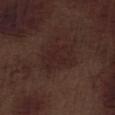| key | value |
|---|---|
| image | total-body-photography crop, ~15 mm field of view |
| patient | male, about 70 years old |
| lesion size | about 4.5 mm |
| site | the leg |